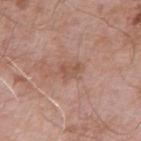The lesion was photographed on a routine skin check and not biopsied; there is no pathology result.
Automated tile analysis of the lesion measured a mean CIELAB color near L≈53 a*≈21 b*≈28 and a normalized lesion–skin contrast near 5.5.
The lesion is located on the right upper arm.
The recorded lesion diameter is about 2.5 mm.
Captured under white-light illumination.
A male patient, roughly 75 years of age.
Cropped from a whole-body photographic skin survey; the tile spans about 15 mm.